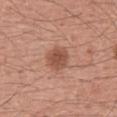Imaged during a routine full-body skin examination; the lesion was not biopsied and no histopathology is available.
The patient is a male aged approximately 45.
From the back.
A 15 mm crop from a total-body photograph taken for skin-cancer surveillance.